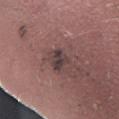Clinical impression:
The lesion was photographed on a routine skin check and not biopsied; there is no pathology result.
Acquisition and patient details:
A roughly 15 mm field-of-view crop from a total-body skin photograph. Longest diameter approximately 2.5 mm. A male subject in their mid-50s. The lesion is on the right forearm. Automated tile analysis of the lesion measured a lesion-detection confidence of about 95/100.